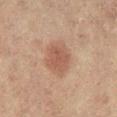patient:
  sex: female
  age_approx: 80
site: leg
image:
  source: total-body photography crop
  field_of_view_mm: 15